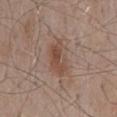Clinical summary:
Located on the mid back. This image is a 15 mm lesion crop taken from a total-body photograph. A male patient roughly 55 years of age.